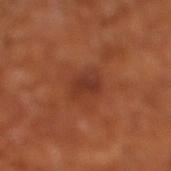Image and clinical context:
Approximately 3.5 mm at its widest. The lesion is on the right lower leg. Cropped from a whole-body photographic skin survey; the tile spans about 15 mm. A subject aged around 65. This is a cross-polarized tile.